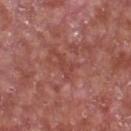Assessment:
Imaged during a routine full-body skin examination; the lesion was not biopsied and no histopathology is available.
Context:
A male subject, roughly 65 years of age. The recorded lesion diameter is about 3 mm. Captured under white-light illumination. A 15 mm close-up extracted from a 3D total-body photography capture. From the chest. The lesion-visualizer software estimated an area of roughly 3 mm², a shape eccentricity near 0.85, and two-axis asymmetry of about 0.65.